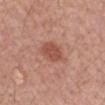No biopsy was performed on this lesion — it was imaged during a full skin examination and was not determined to be concerning.